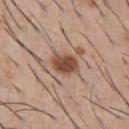Automated image analysis of the tile measured an area of roughly 7.5 mm² and an outline eccentricity of about 0.65 (0 = round, 1 = elongated). The analysis additionally found a mean CIELAB color near L≈49 a*≈20 b*≈29, a lesion–skin lightness drop of about 14, and a lesion-to-skin contrast of about 10 (normalized; higher = more distinct). The software also gave a nevus-likeness score of about 95/100 and a detector confidence of about 100 out of 100 that the crop contains a lesion.
A roughly 15 mm field-of-view crop from a total-body skin photograph.
The lesion's longest dimension is about 3.5 mm.
A male patient, aged approximately 60.
From the front of the torso.